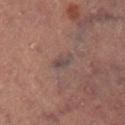This lesion was catalogued during total-body skin photography and was not selected for biopsy. The lesion's longest dimension is about 2.5 mm. The lesion is located on the right lower leg. A lesion tile, about 15 mm wide, cut from a 3D total-body photograph. A female patient aged around 70. This is a cross-polarized tile. An algorithmic analysis of the crop reported a lesion color around L≈36 a*≈13 b*≈15 in CIELAB, roughly 6 lightness units darker than nearby skin, and a normalized border contrast of about 6.5. The analysis additionally found an automated nevus-likeness rating near 0 out of 100.